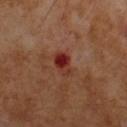notes: no biopsy performed (imaged during a skin exam)
subject: male, in their mid-60s
imaging modality: ~15 mm tile from a whole-body skin photo